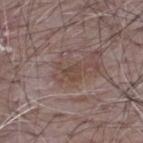Part of a total-body skin-imaging series; this lesion was reviewed on a skin check and was not flagged for biopsy. This is a white-light tile. The patient is a male aged 63–67. Measured at roughly 3 mm in maximum diameter. The lesion is located on the upper back. A region of skin cropped from a whole-body photographic capture, roughly 15 mm wide.